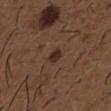Notes:
– notes · imaged on a skin check; not biopsied
– imaging modality · 15 mm crop, total-body photography
– location · the chest
– patient · male, about 50 years old